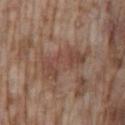This lesion was catalogued during total-body skin photography and was not selected for biopsy.
Cropped from a whole-body photographic skin survey; the tile spans about 15 mm.
This is a white-light tile.
Measured at roughly 6.5 mm in maximum diameter.
A male patient, aged around 75.
The lesion is on the lower back.
Automated image analysis of the tile measured a border-irregularity index near 5/10 and a peripheral color-asymmetry measure near 1.